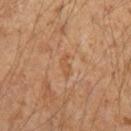| field | value |
|---|---|
| notes | no biopsy performed (imaged during a skin exam) |
| illumination | cross-polarized illumination |
| acquisition | total-body-photography crop, ~15 mm field of view |
| lesion diameter | ~3 mm (longest diameter) |
| automated metrics | a lesion area of about 2.5 mm², a shape eccentricity near 0.95, and a shape-asymmetry score of about 0.5 (0 = symmetric); an average lesion color of about L≈47 a*≈20 b*≈33 (CIELAB), roughly 5 lightness units darker than nearby skin, and a lesion-to-skin contrast of about 5 (normalized; higher = more distinct); a lesion-detection confidence of about 95/100 |
| patient | male, roughly 50 years of age |
| site | the left forearm |